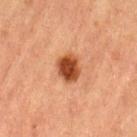follow-up = no biopsy performed (imaged during a skin exam)
automated lesion analysis = a border-irregularity rating of about 1.5/10, a color-variation rating of about 4/10, and radial color variation of about 1; an automated nevus-likeness rating near 100 out of 100 and lesion-presence confidence of about 100/100
tile lighting = cross-polarized illumination
patient = male, aged 83 or over
site = the left thigh
imaging modality = total-body-photography crop, ~15 mm field of view